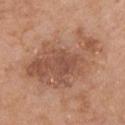<lesion>
  <biopsy_status>not biopsied; imaged during a skin examination</biopsy_status>
  <lighting>white-light</lighting>
  <patient>
    <sex>female</sex>
    <age_approx>65</age_approx>
  </patient>
  <image>
    <source>total-body photography crop</source>
    <field_of_view_mm>15</field_of_view_mm>
  </image>
  <site>chest</site>
  <lesion_size>
    <long_diameter_mm_approx>10.0</long_diameter_mm_approx>
  </lesion_size>
  <automated_metrics>
    <eccentricity>0.8</eccentricity>
    <shape_asymmetry>0.35</shape_asymmetry>
    <vs_skin_darker_L>10.0</vs_skin_darker_L>
    <vs_skin_contrast_norm>7.0</vs_skin_contrast_norm>
    <border_irregularity_0_10>5.5</border_irregularity_0_10>
    <color_variation_0_10>4.5</color_variation_0_10>
    <peripheral_color_asymmetry>1.5</peripheral_color_asymmetry>
  </automated_metrics>
</lesion>